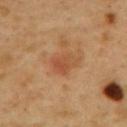| feature | finding |
|---|---|
| biopsy status | imaged on a skin check; not biopsied |
| subject | male, aged around 50 |
| illumination | cross-polarized |
| imaging modality | ~15 mm crop, total-body skin-cancer survey |
| anatomic site | the mid back |
| lesion diameter | ~3.5 mm (longest diameter) |